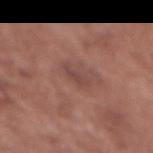The total-body-photography lesion software estimated a lesion area of about 3 mm², a shape eccentricity near 0.9, and a symmetry-axis asymmetry near 0.3. The analysis additionally found about 7 CIELAB-L* units darker than the surrounding skin and a normalized lesion–skin contrast near 5.5. The analysis additionally found a border-irregularity rating of about 3/10, a within-lesion color-variation index near 0.5/10, and a peripheral color-asymmetry measure near 0. And it measured a nevus-likeness score of about 0/100 and a lesion-detection confidence of about 100/100.
Imaged with white-light lighting.
The patient is a female in their mid- to late 70s.
Measured at roughly 2.5 mm in maximum diameter.
Located on the chest.
A region of skin cropped from a whole-body photographic capture, roughly 15 mm wide.